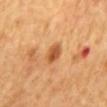• follow-up — imaged on a skin check; not biopsied
• image source — total-body-photography crop, ~15 mm field of view
• patient — male, about 65 years old
• body site — the back